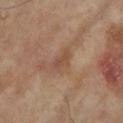Captured during whole-body skin photography for melanoma surveillance; the lesion was not biopsied. The lesion's longest dimension is about 3 mm. A lesion tile, about 15 mm wide, cut from a 3D total-body photograph. From the right lower leg. The tile uses cross-polarized illumination. A female patient, aged around 75.